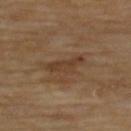follow-up = total-body-photography surveillance lesion; no biopsy
body site = the back
imaging modality = ~15 mm crop, total-body skin-cancer survey
subject = male, aged 68 to 72
lesion diameter = ≈4.5 mm